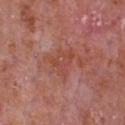| key | value |
|---|---|
| follow-up | no biopsy performed (imaged during a skin exam) |
| diameter | ~3.5 mm (longest diameter) |
| tile lighting | white-light illumination |
| site | the chest |
| patient | male, aged 63 to 67 |
| imaging modality | ~15 mm tile from a whole-body skin photo |
| automated lesion analysis | a border-irregularity rating of about 5/10 and a within-lesion color-variation index near 3.5/10; an automated nevus-likeness rating near 0 out of 100 and lesion-presence confidence of about 100/100 |